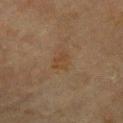Impression:
Part of a total-body skin-imaging series; this lesion was reviewed on a skin check and was not flagged for biopsy.
Image and clinical context:
The lesion is on the left upper arm. Captured under cross-polarized illumination. A lesion tile, about 15 mm wide, cut from a 3D total-body photograph. The total-body-photography lesion software estimated a mean CIELAB color near L≈34 a*≈14 b*≈27, about 4 CIELAB-L* units darker than the surrounding skin, and a lesion-to-skin contrast of about 6 (normalized; higher = more distinct). And it measured a border-irregularity index near 3.5/10 and a within-lesion color-variation index near 1.5/10. The software also gave a nevus-likeness score of about 5/100. A male patient in their 70s. About 3 mm across.